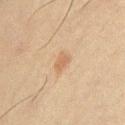Q: How was this image acquired?
A: 15 mm crop, total-body photography
Q: Illumination type?
A: cross-polarized
Q: Lesion location?
A: the chest
Q: Patient demographics?
A: male, roughly 60 years of age